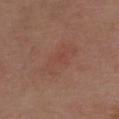The lesion was photographed on a routine skin check and not biopsied; there is no pathology result. The tile uses white-light illumination. About 5.5 mm across. A 15 mm close-up tile from a total-body photography series done for melanoma screening. On the upper back. A male patient, roughly 70 years of age. Automated tile analysis of the lesion measured an average lesion color of about L≈46 a*≈22 b*≈26 (CIELAB), roughly 4 lightness units darker than nearby skin, and a lesion-to-skin contrast of about 3.5 (normalized; higher = more distinct). And it measured an automated nevus-likeness rating near 5 out of 100 and a detector confidence of about 100 out of 100 that the crop contains a lesion.